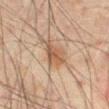{"biopsy_status": "not biopsied; imaged during a skin examination", "automated_metrics": {"area_mm2_approx": 7.5, "eccentricity": 0.65, "cielab_L": 46, "cielab_a": 16, "cielab_b": 28, "vs_skin_darker_L": 8.0, "border_irregularity_0_10": 3.5, "color_variation_0_10": 3.5, "peripheral_color_asymmetry": 1.0, "nevus_likeness_0_100": 25}, "lighting": "cross-polarized", "image": {"source": "total-body photography crop", "field_of_view_mm": 15}, "patient": {"sex": "male", "age_approx": 70}, "lesion_size": {"long_diameter_mm_approx": 3.5}, "site": "mid back"}